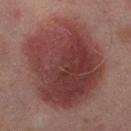Recorded during total-body skin imaging; not selected for excision or biopsy.
Cropped from a total-body skin-imaging series; the visible field is about 15 mm.
The subject is a female in their 50s.
Automated tile analysis of the lesion measured an average lesion color of about L≈38 a*≈23 b*≈21 (CIELAB) and a lesion-to-skin contrast of about 9.5 (normalized; higher = more distinct). The analysis additionally found border irregularity of about 2 on a 0–10 scale, internal color variation of about 6.5 on a 0–10 scale, and peripheral color asymmetry of about 2.5. The analysis additionally found an automated nevus-likeness rating near 100 out of 100.
On the left thigh.
Imaged with cross-polarized lighting.